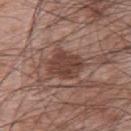biopsy_status: not biopsied; imaged during a skin examination
site: upper back
lesion_size:
  long_diameter_mm_approx: 4.5
image:
  source: total-body photography crop
  field_of_view_mm: 15
patient:
  sex: male
  age_approx: 65
lighting: white-light